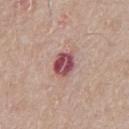* follow-up — catalogued during a skin exam; not biopsied
* patient — male, in their mid- to late 60s
* image — ~15 mm crop, total-body skin-cancer survey
* site — the chest